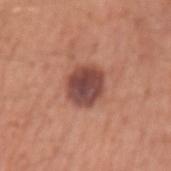Q: What are the patient's age and sex?
A: male, in their 60s
Q: What is the anatomic site?
A: the arm
Q: Illumination type?
A: white-light
Q: How was this image acquired?
A: 15 mm crop, total-body photography